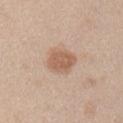Q: What are the patient's age and sex?
A: male, approximately 45 years of age
Q: How was this image acquired?
A: ~15 mm crop, total-body skin-cancer survey
Q: What did automated image analysis measure?
A: a classifier nevus-likeness of about 90/100 and lesion-presence confidence of about 100/100
Q: What is the lesion's diameter?
A: ~3.5 mm (longest diameter)
Q: What is the anatomic site?
A: the right upper arm